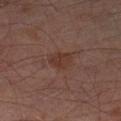Part of a total-body skin-imaging series; this lesion was reviewed on a skin check and was not flagged for biopsy. From the left thigh. A 15 mm crop from a total-body photograph taken for skin-cancer surveillance. The total-body-photography lesion software estimated a border-irregularity rating of about 2/10 and a color-variation rating of about 2/10. The software also gave a nevus-likeness score of about 5/100 and lesion-presence confidence of about 100/100. This is a cross-polarized tile. A male patient, roughly 65 years of age.